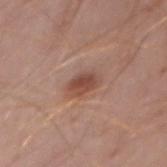Clinical impression:
This lesion was catalogued during total-body skin photography and was not selected for biopsy.
Clinical summary:
The recorded lesion diameter is about 3.5 mm. A male patient, approximately 40 years of age. The lesion is located on the abdomen. A 15 mm close-up extracted from a 3D total-body photography capture. Captured under white-light illumination. Automated image analysis of the tile measured an average lesion color of about L≈48 a*≈22 b*≈27 (CIELAB), roughly 11 lightness units darker than nearby skin, and a normalized lesion–skin contrast near 8.